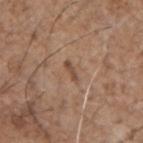Case summary:
• workup: total-body-photography surveillance lesion; no biopsy
• imaging modality: ~15 mm tile from a whole-body skin photo
• subject: male, aged 68–72
• automated lesion analysis: a border-irregularity rating of about 3/10, internal color variation of about 0 on a 0–10 scale, and a peripheral color-asymmetry measure near 0; an automated nevus-likeness rating near 0 out of 100 and lesion-presence confidence of about 95/100
• size: about 2.5 mm
• body site: the arm
• tile lighting: white-light illumination